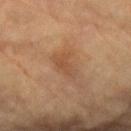follow-up=no biopsy performed (imaged during a skin exam)
diameter=~3.5 mm (longest diameter)
acquisition=~15 mm crop, total-body skin-cancer survey
lighting=cross-polarized illumination
anatomic site=the left forearm
subject=male, aged approximately 85 or older